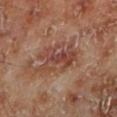- notes: imaged on a skin check; not biopsied
- body site: the left lower leg
- image source: ~15 mm crop, total-body skin-cancer survey
- lighting: cross-polarized illumination
- TBP lesion metrics: an area of roughly 14 mm² and an outline eccentricity of about 0.7 (0 = round, 1 = elongated)
- diameter: about 5 mm
- subject: male, aged 68–72